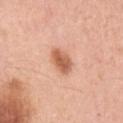Clinical impression: The lesion was tiled from a total-body skin photograph and was not biopsied. Clinical summary: A 15 mm crop from a total-body photograph taken for skin-cancer surveillance. Approximately 3 mm at its widest. The patient is a female aged 38–42. The total-body-photography lesion software estimated border irregularity of about 1.5 on a 0–10 scale, internal color variation of about 3.5 on a 0–10 scale, and radial color variation of about 1. The software also gave an automated nevus-likeness rating near 95 out of 100 and a detector confidence of about 100 out of 100 that the crop contains a lesion. From the arm.